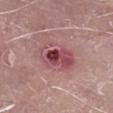The lesion was photographed on a routine skin check and not biopsied; there is no pathology result. From the leg. The lesion's longest dimension is about 5 mm. This image is a 15 mm lesion crop taken from a total-body photograph. A male subject, in their mid-60s.